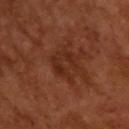Clinical summary:
A 15 mm close-up extracted from a 3D total-body photography capture. A male patient about 65 years old.